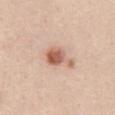Impression: Captured during whole-body skin photography for melanoma surveillance; the lesion was not biopsied. Clinical summary: A 15 mm close-up tile from a total-body photography series done for melanoma screening. The patient is a female about 45 years old. Located on the abdomen. An algorithmic analysis of the crop reported an average lesion color of about L≈60 a*≈23 b*≈30 (CIELAB), about 14 CIELAB-L* units darker than the surrounding skin, and a normalized border contrast of about 9. The analysis additionally found a within-lesion color-variation index near 4.5/10 and peripheral color asymmetry of about 1.5. It also reported a classifier nevus-likeness of about 95/100 and a lesion-detection confidence of about 100/100. The lesion's longest dimension is about 4 mm. Captured under white-light illumination.